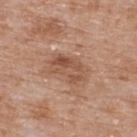A female subject roughly 75 years of age. Located on the upper back. Approximately 4.5 mm at its widest. Captured under white-light illumination. A 15 mm close-up tile from a total-body photography series done for melanoma screening.